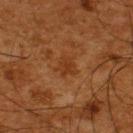Q: Was a biopsy performed?
A: total-body-photography surveillance lesion; no biopsy
Q: What is the lesion's diameter?
A: ~2.5 mm (longest diameter)
Q: What are the patient's age and sex?
A: male, about 65 years old
Q: What is the imaging modality?
A: ~15 mm tile from a whole-body skin photo
Q: Where on the body is the lesion?
A: the upper back